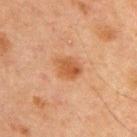Case summary:
– follow-up: no biopsy performed (imaged during a skin exam)
– illumination: cross-polarized
– image-analysis metrics: an area of roughly 6 mm², an outline eccentricity of about 0.65 (0 = round, 1 = elongated), and two-axis asymmetry of about 0.2; a border-irregularity rating of about 2/10 and a peripheral color-asymmetry measure near 1; a classifier nevus-likeness of about 75/100 and a detector confidence of about 100 out of 100 that the crop contains a lesion
– site: the chest
– acquisition: total-body-photography crop, ~15 mm field of view
– lesion diameter: ~3 mm (longest diameter)
– patient: male, in their mid-60s